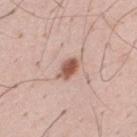This lesion was catalogued during total-body skin photography and was not selected for biopsy. The total-body-photography lesion software estimated an eccentricity of roughly 0.8 and two-axis asymmetry of about 0.25. It also reported a lesion color around L≈55 a*≈23 b*≈27 in CIELAB and a lesion–skin lightness drop of about 15. It also reported a border-irregularity index near 2.5/10, a color-variation rating of about 3.5/10, and radial color variation of about 1. The tile uses white-light illumination. From the mid back. Longest diameter approximately 3 mm. A male patient, in their mid-30s. A 15 mm close-up extracted from a 3D total-body photography capture.